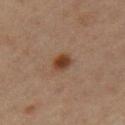Part of a total-body skin-imaging series; this lesion was reviewed on a skin check and was not flagged for biopsy.
A close-up tile cropped from a whole-body skin photograph, about 15 mm across.
The subject is a female aged 43–47.
Measured at roughly 2.5 mm in maximum diameter.
On the arm.
The total-body-photography lesion software estimated internal color variation of about 4 on a 0–10 scale and radial color variation of about 1.5.
This is a cross-polarized tile.